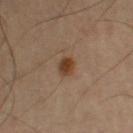follow-up = catalogued during a skin exam; not biopsied | size = about 2.5 mm | location = the right upper arm | acquisition = ~15 mm tile from a whole-body skin photo | patient = male, aged 63–67 | image-analysis metrics = an area of roughly 4 mm², an eccentricity of roughly 0.65, and two-axis asymmetry of about 0.2; an automated nevus-likeness rating near 100 out of 100 and a detector confidence of about 100 out of 100 that the crop contains a lesion.